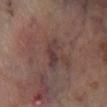{"biopsy_status": "not biopsied; imaged during a skin examination", "image": {"source": "total-body photography crop", "field_of_view_mm": 15}, "lighting": "cross-polarized", "patient": {"sex": "male", "age_approx": 70}, "lesion_size": {"long_diameter_mm_approx": 4.0}, "site": "right lower leg"}